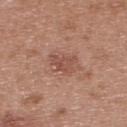notes: imaged on a skin check; not biopsied | diameter: about 3.5 mm | image source: total-body-photography crop, ~15 mm field of view | subject: female, aged approximately 40 | lighting: white-light illumination | automated metrics: a lesion area of about 5.5 mm², an outline eccentricity of about 0.75 (0 = round, 1 = elongated), and a symmetry-axis asymmetry near 0.45; border irregularity of about 4.5 on a 0–10 scale, internal color variation of about 2.5 on a 0–10 scale, and radial color variation of about 1; a classifier nevus-likeness of about 0/100 and lesion-presence confidence of about 100/100 | anatomic site: the upper back.